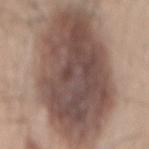Imaged during a routine full-body skin examination; the lesion was not biopsied and no histopathology is available.
Captured under white-light illumination.
A male patient, aged around 45.
The lesion is located on the lower back.
An algorithmic analysis of the crop reported a lesion color around L≈49 a*≈16 b*≈22 in CIELAB, roughly 16 lightness units darker than nearby skin, and a normalized border contrast of about 11.5. The software also gave border irregularity of about 2.5 on a 0–10 scale.
A 15 mm close-up extracted from a 3D total-body photography capture.
The lesion's longest dimension is about 16.5 mm.